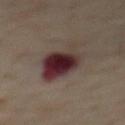No biopsy was performed on this lesion — it was imaged during a full skin examination and was not determined to be concerning. Imaged with cross-polarized lighting. A roughly 15 mm field-of-view crop from a total-body skin photograph. The lesion is on the chest. The subject is a male aged 63–67.